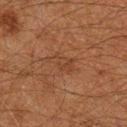biopsy_status: not biopsied; imaged during a skin examination
site: leg
image:
  source: total-body photography crop
  field_of_view_mm: 15
lighting: cross-polarized
lesion_size:
  long_diameter_mm_approx: 3.5
patient:
  sex: male
  age_approx: 60
automated_metrics:
  area_mm2_approx: 5.0
  eccentricity: 0.85
  cielab_L: 31
  cielab_a: 17
  cielab_b: 25
  vs_skin_contrast_norm: 4.5
  lesion_detection_confidence_0_100: 100